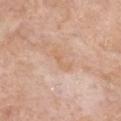Q: Is there a histopathology result?
A: total-body-photography surveillance lesion; no biopsy
Q: Who is the patient?
A: female, aged 73 to 77
Q: What is the anatomic site?
A: the front of the torso
Q: Lesion size?
A: ~3.5 mm (longest diameter)
Q: How was this image acquired?
A: 15 mm crop, total-body photography